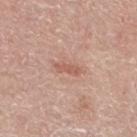| feature | finding |
|---|---|
| notes | catalogued during a skin exam; not biopsied |
| acquisition | ~15 mm tile from a whole-body skin photo |
| body site | the left lower leg |
| patient | male, about 70 years old |
| automated lesion analysis | an average lesion color of about L≈58 a*≈23 b*≈28 (CIELAB), about 9 CIELAB-L* units darker than the surrounding skin, and a lesion-to-skin contrast of about 6 (normalized; higher = more distinct); internal color variation of about 0 on a 0–10 scale and peripheral color asymmetry of about 0; an automated nevus-likeness rating near 0 out of 100 and a lesion-detection confidence of about 100/100 |
| tile lighting | white-light |
| lesion size | ≈3 mm |